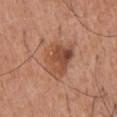Findings:
* follow-up: imaged on a skin check; not biopsied
* location: the upper back
* patient: male, aged 53 to 57
* imaging modality: ~15 mm tile from a whole-body skin photo